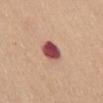Impression:
No biopsy was performed on this lesion — it was imaged during a full skin examination and was not determined to be concerning.
Acquisition and patient details:
Captured under white-light illumination. Longest diameter approximately 3 mm. A region of skin cropped from a whole-body photographic capture, roughly 15 mm wide. A female patient roughly 60 years of age. Located on the abdomen.